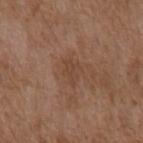• follow-up — total-body-photography surveillance lesion; no biopsy